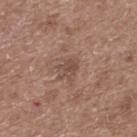<tbp_lesion>
<biopsy_status>not biopsied; imaged during a skin examination</biopsy_status>
<patient>
  <sex>male</sex>
  <age_approx>60</age_approx>
</patient>
<site>upper back</site>
<lighting>white-light</lighting>
<lesion_size>
  <long_diameter_mm_approx>3.0</long_diameter_mm_approx>
</lesion_size>
<image>
  <source>total-body photography crop</source>
  <field_of_view_mm>15</field_of_view_mm>
</image>
</tbp_lesion>